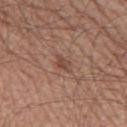Captured during whole-body skin photography for melanoma surveillance; the lesion was not biopsied. A close-up tile cropped from a whole-body skin photograph, about 15 mm across. On the left thigh. A male patient, aged around 60.